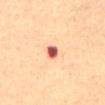No biopsy was performed on this lesion — it was imaged during a full skin examination and was not determined to be concerning.